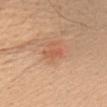No biopsy was performed on this lesion — it was imaged during a full skin examination and was not determined to be concerning.
The total-body-photography lesion software estimated an outline eccentricity of about 0.9 (0 = round, 1 = elongated) and a shape-asymmetry score of about 0.3 (0 = symmetric). The analysis additionally found an average lesion color of about L≈57 a*≈27 b*≈34 (CIELAB), roughly 7 lightness units darker than nearby skin, and a normalized lesion–skin contrast near 5.
A 15 mm close-up tile from a total-body photography series done for melanoma screening.
Imaged with white-light lighting.
A male patient, roughly 60 years of age.
The lesion is on the chest.